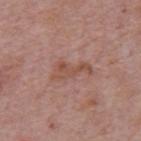{
  "biopsy_status": "not biopsied; imaged during a skin examination",
  "site": "back",
  "image": {
    "source": "total-body photography crop",
    "field_of_view_mm": 15
  },
  "patient": {
    "sex": "male",
    "age_approx": 75
  }
}